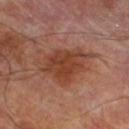Measured at roughly 7 mm in maximum diameter. A 15 mm crop from a total-body photograph taken for skin-cancer surveillance. A male subject, about 70 years old. Automated tile analysis of the lesion measured a shape eccentricity near 0.75 and a symmetry-axis asymmetry near 0.3. And it measured a mean CIELAB color near L≈41 a*≈23 b*≈29, a lesion–skin lightness drop of about 10, and a normalized lesion–skin contrast near 8.5. The lesion is located on the left lower leg. Captured under cross-polarized illumination.